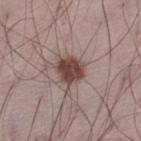Part of a total-body skin-imaging series; this lesion was reviewed on a skin check and was not flagged for biopsy.
The lesion's longest dimension is about 3 mm.
Cropped from a total-body skin-imaging series; the visible field is about 15 mm.
The lesion is on the left thigh.
Automated image analysis of the tile measured a lesion area of about 7.5 mm², an eccentricity of roughly 0.5, and two-axis asymmetry of about 0.2. And it measured a border-irregularity rating of about 2/10, internal color variation of about 3 on a 0–10 scale, and peripheral color asymmetry of about 1.
A male subject aged 68 to 72.
Imaged with white-light lighting.